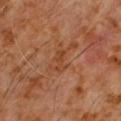Case summary:
• imaging modality: ~15 mm tile from a whole-body skin photo
• subject: male, aged 58 to 62
• location: the chest
• illumination: cross-polarized
• lesion diameter: ~3 mm (longest diameter)